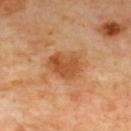Case summary:
- notes: no biopsy performed (imaged during a skin exam)
- size: ≈4.5 mm
- tile lighting: cross-polarized
- acquisition: total-body-photography crop, ~15 mm field of view
- site: the upper back